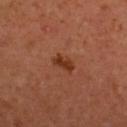Clinical summary: A female patient aged 38–42. This image is a 15 mm lesion crop taken from a total-body photograph. Captured under cross-polarized illumination. From the upper back. The total-body-photography lesion software estimated a lesion area of about 4 mm² and a symmetry-axis asymmetry near 0.3. The analysis additionally found a border-irregularity index near 3.5/10, a color-variation rating of about 2/10, and radial color variation of about 1.